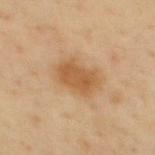This lesion was catalogued during total-body skin photography and was not selected for biopsy.
Measured at roughly 5 mm in maximum diameter.
Imaged with cross-polarized lighting.
The subject is a male aged 38 to 42.
A 15 mm close-up extracted from a 3D total-body photography capture.
From the back.